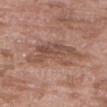notes=no biopsy performed (imaged during a skin exam); imaging modality=15 mm crop, total-body photography; anatomic site=the right upper arm; size=~6.5 mm (longest diameter); illumination=white-light illumination; subject=female, aged 68–72.